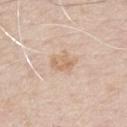site: the chest
image: ~15 mm tile from a whole-body skin photo
patient: male, aged 68–72